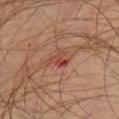No biopsy was performed on this lesion — it was imaged during a full skin examination and was not determined to be concerning.
Approximately 2.5 mm at its widest.
A 15 mm crop from a total-body photograph taken for skin-cancer surveillance.
An algorithmic analysis of the crop reported an area of roughly 4 mm², an eccentricity of roughly 0.65, and a shape-asymmetry score of about 0.4 (0 = symmetric).
The patient is a male aged 28 to 32.
The lesion is located on the chest.